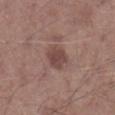On the right lower leg.
The subject is a male aged 58 to 62.
A lesion tile, about 15 mm wide, cut from a 3D total-body photograph.
An algorithmic analysis of the crop reported an average lesion color of about L≈45 a*≈19 b*≈21 (CIELAB), about 9 CIELAB-L* units darker than the surrounding skin, and a lesion-to-skin contrast of about 7.5 (normalized; higher = more distinct). The analysis additionally found a nevus-likeness score of about 40/100 and a lesion-detection confidence of about 100/100.